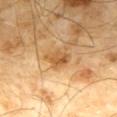Part of a total-body skin-imaging series; this lesion was reviewed on a skin check and was not flagged for biopsy. The lesion is located on the mid back. A male patient aged approximately 65. A lesion tile, about 15 mm wide, cut from a 3D total-body photograph.